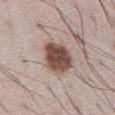The lesion was photographed on a routine skin check and not biopsied; there is no pathology result.